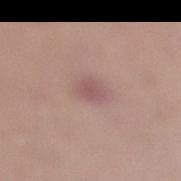Recorded during total-body skin imaging; not selected for excision or biopsy.
A female subject roughly 20 years of age.
Located on the right lower leg.
This image is a 15 mm lesion crop taken from a total-body photograph.
This is a white-light tile.